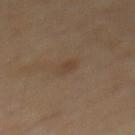<case>
  <biopsy_status>not biopsied; imaged during a skin examination</biopsy_status>
  <site>back</site>
  <patient>
    <sex>male</sex>
    <age_approx>70</age_approx>
  </patient>
  <image>
    <source>total-body photography crop</source>
    <field_of_view_mm>15</field_of_view_mm>
  </image>
</case>